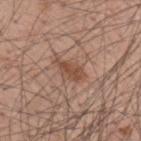– biopsy status — catalogued during a skin exam; not biopsied
– image — ~15 mm crop, total-body skin-cancer survey
– body site — the mid back
– subject — male, aged 58 to 62
– tile lighting — white-light
– lesion size — ≈3.5 mm